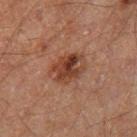The lesion was tiled from a total-body skin photograph and was not biopsied.
A lesion tile, about 15 mm wide, cut from a 3D total-body photograph.
On the right thigh.
Imaged with cross-polarized lighting.
About 4 mm across.
The subject is a male aged 58 to 62.
An algorithmic analysis of the crop reported an area of roughly 8 mm² and an eccentricity of roughly 0.6. The software also gave an average lesion color of about L≈29 a*≈19 b*≈24 (CIELAB), roughly 9 lightness units darker than nearby skin, and a normalized lesion–skin contrast near 9. It also reported a border-irregularity rating of about 3.5/10, internal color variation of about 5 on a 0–10 scale, and radial color variation of about 2.